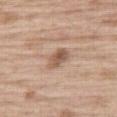No biopsy was performed on this lesion — it was imaged during a full skin examination and was not determined to be concerning.
A 15 mm crop from a total-body photograph taken for skin-cancer surveillance.
A male subject, aged around 70.
The lesion is on the right thigh.